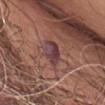The lesion was tiled from a total-body skin photograph and was not biopsied.
On the abdomen.
A male subject in their 60s.
The lesion-visualizer software estimated a footprint of about 6.5 mm², an eccentricity of roughly 0.8, and a symmetry-axis asymmetry near 0.15. The analysis additionally found an average lesion color of about L≈40 a*≈22 b*≈17 (CIELAB), about 10 CIELAB-L* units darker than the surrounding skin, and a normalized border contrast of about 10.5. It also reported a nevus-likeness score of about 0/100 and a lesion-detection confidence of about 90/100.
Captured under white-light illumination.
A close-up tile cropped from a whole-body skin photograph, about 15 mm across.